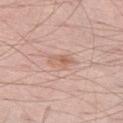size: ≈3.5 mm | patient: male, about 55 years old | anatomic site: the left thigh | acquisition: 15 mm crop, total-body photography.